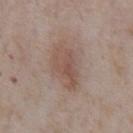Clinical impression:
Captured during whole-body skin photography for melanoma surveillance; the lesion was not biopsied.
Background:
A 15 mm close-up extracted from a 3D total-body photography capture. On the chest. The subject is a male aged approximately 75.